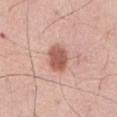  biopsy_status: not biopsied; imaged during a skin examination
  automated_metrics:
    cielab_L: 57
    cielab_a: 24
    cielab_b: 28
    vs_skin_contrast_norm: 9.0
    border_irregularity_0_10: 1.5
    color_variation_0_10: 3.0
    peripheral_color_asymmetry: 1.0
  lesion_size:
    long_diameter_mm_approx: 3.5
  site: abdomen
  patient:
    sex: male
    age_approx: 70
  image:
    source: total-body photography crop
    field_of_view_mm: 15